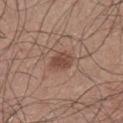| feature | finding |
|---|---|
| notes | no biopsy performed (imaged during a skin exam) |
| acquisition | ~15 mm crop, total-body skin-cancer survey |
| lesion size | about 3 mm |
| patient | male, in their mid-60s |
| image-analysis metrics | a lesion area of about 5.5 mm², a shape eccentricity near 0.75, and a shape-asymmetry score of about 0.25 (0 = symmetric); an average lesion color of about L≈46 a*≈20 b*≈26 (CIELAB) and a normalized border contrast of about 7.5; border irregularity of about 2 on a 0–10 scale, a within-lesion color-variation index near 2/10, and a peripheral color-asymmetry measure near 0.5 |
| tile lighting | white-light |
| location | the left lower leg |